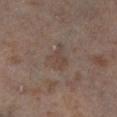Clinical summary: Automated image analysis of the tile measured a footprint of about 5 mm², an eccentricity of roughly 0.85, and a symmetry-axis asymmetry near 0.4. And it measured a mean CIELAB color near L≈40 a*≈14 b*≈21 and a normalized lesion–skin contrast near 5. The software also gave a border-irregularity rating of about 5.5/10 and radial color variation of about 0.5. Captured under cross-polarized illumination. A female patient aged approximately 50. On the left lower leg. The recorded lesion diameter is about 3.5 mm. This image is a 15 mm lesion crop taken from a total-body photograph.